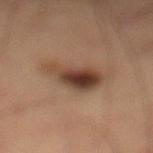Assessment:
Imaged during a routine full-body skin examination; the lesion was not biopsied and no histopathology is available.
Background:
A male subject, aged around 65. The lesion is on the left lower leg. This image is a 15 mm lesion crop taken from a total-body photograph.